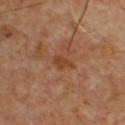Imaged during a routine full-body skin examination; the lesion was not biopsied and no histopathology is available. From the chest. The subject is a male about 50 years old. Captured under cross-polarized illumination. Cropped from a total-body skin-imaging series; the visible field is about 15 mm. An algorithmic analysis of the crop reported an area of roughly 3 mm², a shape eccentricity near 0.85, and two-axis asymmetry of about 0.3. It also reported a border-irregularity index near 3/10, internal color variation of about 1 on a 0–10 scale, and peripheral color asymmetry of about 0.5. It also reported an automated nevus-likeness rating near 0 out of 100 and a lesion-detection confidence of about 100/100.